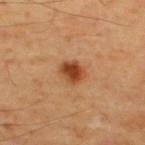{
  "biopsy_status": "not biopsied; imaged during a skin examination",
  "automated_metrics": {
    "cielab_L": 44,
    "cielab_a": 26,
    "cielab_b": 38,
    "vs_skin_contrast_norm": 10.5,
    "nevus_likeness_0_100": 100,
    "lesion_detection_confidence_0_100": 100
  },
  "patient": {
    "sex": "male",
    "age_approx": 55
  },
  "image": {
    "source": "total-body photography crop",
    "field_of_view_mm": 15
  },
  "site": "upper back",
  "lighting": "cross-polarized",
  "lesion_size": {
    "long_diameter_mm_approx": 3.0
  }
}